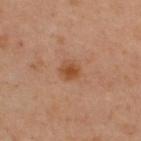Imaged during a routine full-body skin examination; the lesion was not biopsied and no histopathology is available. This is a cross-polarized tile. The subject is a male aged 43–47. On the upper back. This image is a 15 mm lesion crop taken from a total-body photograph.